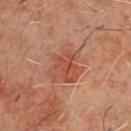The lesion was tiled from a total-body skin photograph and was not biopsied. On the chest. A male subject, aged around 60. An algorithmic analysis of the crop reported an average lesion color of about L≈47 a*≈25 b*≈30 (CIELAB), about 7 CIELAB-L* units darker than the surrounding skin, and a normalized border contrast of about 5.5. The analysis additionally found an automated nevus-likeness rating near 5 out of 100 and a lesion-detection confidence of about 100/100. Captured under cross-polarized illumination. This image is a 15 mm lesion crop taken from a total-body photograph.